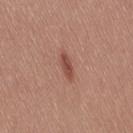| field | value |
|---|---|
| workup | no biopsy performed (imaged during a skin exam) |
| lighting | white-light |
| automated metrics | an outline eccentricity of about 0.9 (0 = round, 1 = elongated) and a shape-asymmetry score of about 0.3 (0 = symmetric); a lesion color around L≈48 a*≈25 b*≈27 in CIELAB, a lesion–skin lightness drop of about 11, and a normalized lesion–skin contrast near 8; a border-irregularity rating of about 3/10, a within-lesion color-variation index near 0.5/10, and a peripheral color-asymmetry measure near 0 |
| anatomic site | the right thigh |
| lesion diameter | ≈3 mm |
| patient | female, aged 23 to 27 |
| image source | ~15 mm tile from a whole-body skin photo |